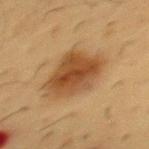biopsy status: no biopsy performed (imaged during a skin exam)
patient: male, aged 53–57
automated metrics: an outline eccentricity of about 0.65 (0 = round, 1 = elongated) and two-axis asymmetry of about 0.25; about 11 CIELAB-L* units darker than the surrounding skin and a normalized lesion–skin contrast near 9; a border-irregularity rating of about 2.5/10, a color-variation rating of about 5/10, and radial color variation of about 1.5; a detector confidence of about 100 out of 100 that the crop contains a lesion
tile lighting: cross-polarized illumination
image: ~15 mm tile from a whole-body skin photo
body site: the chest
size: ≈6 mm